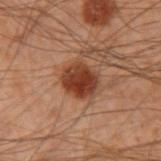workup=total-body-photography surveillance lesion; no biopsy | subject=male, aged 48 to 52 | site=the left forearm | image=~15 mm crop, total-body skin-cancer survey.